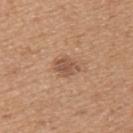This lesion was catalogued during total-body skin photography and was not selected for biopsy. The patient is a male aged around 40. About 3 mm across. Imaged with white-light lighting. On the upper back. A roughly 15 mm field-of-view crop from a total-body skin photograph.